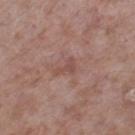biopsy status = total-body-photography surveillance lesion; no biopsy | subject = male, roughly 55 years of age | body site = the right lower leg | imaging modality = ~15 mm crop, total-body skin-cancer survey.